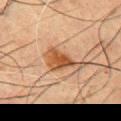The lesion was photographed on a routine skin check and not biopsied; there is no pathology result.
Automated tile analysis of the lesion measured a symmetry-axis asymmetry near 0.5. The analysis additionally found a mean CIELAB color near L≈42 a*≈19 b*≈31 and about 10 CIELAB-L* units darker than the surrounding skin. And it measured a border-irregularity index near 5.5/10 and a peripheral color-asymmetry measure near 1.
On the chest.
About 4.5 mm across.
The tile uses cross-polarized illumination.
A male subject aged 48 to 52.
Cropped from a whole-body photographic skin survey; the tile spans about 15 mm.